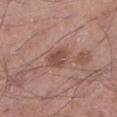Imaged during a routine full-body skin examination; the lesion was not biopsied and no histopathology is available. About 3 mm across. Captured under white-light illumination. A male patient in their mid-50s. Automated image analysis of the tile measured a border-irregularity rating of about 2.5/10 and internal color variation of about 2 on a 0–10 scale. The analysis additionally found a nevus-likeness score of about 75/100 and a lesion-detection confidence of about 100/100. Located on the left lower leg. A roughly 15 mm field-of-view crop from a total-body skin photograph.